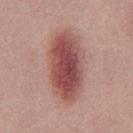Clinical impression: Part of a total-body skin-imaging series; this lesion was reviewed on a skin check and was not flagged for biopsy. Context: Imaged with white-light lighting. The patient is a male roughly 30 years of age. The lesion-visualizer software estimated a lesion area of about 28 mm², an outline eccentricity of about 0.85 (0 = round, 1 = elongated), and a symmetry-axis asymmetry near 0.05. The software also gave a border-irregularity index near 1.5/10, internal color variation of about 7 on a 0–10 scale, and radial color variation of about 2. It also reported lesion-presence confidence of about 100/100. A 15 mm close-up extracted from a 3D total-body photography capture.